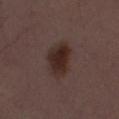This lesion was catalogued during total-body skin photography and was not selected for biopsy.